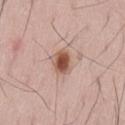Clinical impression:
Captured during whole-body skin photography for melanoma surveillance; the lesion was not biopsied.
Context:
The lesion is located on the lower back. A male subject, aged approximately 65. Automated tile analysis of the lesion measured a border-irregularity index near 1.5/10, a color-variation rating of about 5/10, and radial color variation of about 1.5. The recorded lesion diameter is about 3 mm. A 15 mm close-up tile from a total-body photography series done for melanoma screening.